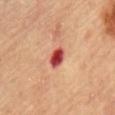Context: Located on the chest. Approximately 3 mm at its widest. The patient is a male roughly 85 years of age or older. Cropped from a total-body skin-imaging series; the visible field is about 15 mm. Imaged with cross-polarized lighting. The total-body-photography lesion software estimated a border-irregularity index near 2.5/10 and peripheral color asymmetry of about 2.